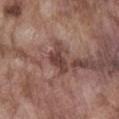Clinical impression:
No biopsy was performed on this lesion — it was imaged during a full skin examination and was not determined to be concerning.
Image and clinical context:
The lesion is on the abdomen. This is a white-light tile. A 15 mm close-up extracted from a 3D total-body photography capture. A male patient, aged 73 to 77. Approximately 4 mm at its widest.